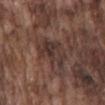| key | value |
|---|---|
| workup | catalogued during a skin exam; not biopsied |
| image source | 15 mm crop, total-body photography |
| automated metrics | a symmetry-axis asymmetry near 0.35 |
| size | ~5 mm (longest diameter) |
| patient | male, aged 73 to 77 |
| tile lighting | white-light illumination |
| location | the mid back |